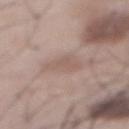Impression:
This lesion was catalogued during total-body skin photography and was not selected for biopsy.
Background:
A male subject, approximately 55 years of age. A 15 mm close-up tile from a total-body photography series done for melanoma screening. The lesion's longest dimension is about 3.5 mm. From the abdomen. Imaged with white-light lighting.